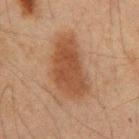This lesion was catalogued during total-body skin photography and was not selected for biopsy.
The total-body-photography lesion software estimated an average lesion color of about L≈40 a*≈19 b*≈29 (CIELAB), a lesion–skin lightness drop of about 9, and a lesion-to-skin contrast of about 8 (normalized; higher = more distinct). The analysis additionally found a border-irregularity rating of about 3.5/10. The analysis additionally found a nevus-likeness score of about 100/100 and lesion-presence confidence of about 100/100.
A lesion tile, about 15 mm wide, cut from a 3D total-body photograph.
The lesion's longest dimension is about 7.5 mm.
The patient is a male in their mid-60s.
From the mid back.
Imaged with cross-polarized lighting.